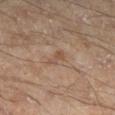tile lighting: cross-polarized illumination
image source: total-body-photography crop, ~15 mm field of view
automated metrics: a border-irregularity index near 6/10, internal color variation of about 0 on a 0–10 scale, and a peripheral color-asymmetry measure near 0; a nevus-likeness score of about 0/100 and a lesion-detection confidence of about 100/100
location: the right lower leg
patient: male, aged approximately 45